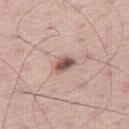A male patient, roughly 60 years of age. A region of skin cropped from a whole-body photographic capture, roughly 15 mm wide. The total-body-photography lesion software estimated a detector confidence of about 100 out of 100 that the crop contains a lesion. The lesion is on the leg.